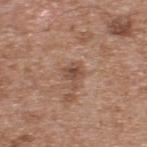Q: Was a biopsy performed?
A: no biopsy performed (imaged during a skin exam)
Q: Automated lesion metrics?
A: a lesion area of about 4 mm² and a shape eccentricity near 0.6; a nevus-likeness score of about 0/100
Q: What is the imaging modality?
A: ~15 mm tile from a whole-body skin photo
Q: Where on the body is the lesion?
A: the upper back
Q: How large is the lesion?
A: ≈2.5 mm
Q: What lighting was used for the tile?
A: white-light
Q: Who is the patient?
A: male, aged around 65